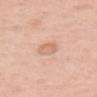automated lesion analysis = a border-irregularity rating of about 2/10 and peripheral color asymmetry of about 0.5; an automated nevus-likeness rating near 65 out of 100 | site = the back | lighting = white-light | diameter = about 3 mm | subject = female, aged around 50 | imaging modality = ~15 mm crop, total-body skin-cancer survey.